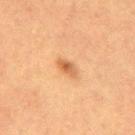Notes:
– imaging modality · ~15 mm crop, total-body skin-cancer survey
– automated metrics · a footprint of about 3 mm², a shape eccentricity near 0.9, and a shape-asymmetry score of about 0.4 (0 = symmetric); an average lesion color of about L≈49 a*≈21 b*≈34 (CIELAB) and roughly 10 lightness units darker than nearby skin; a color-variation rating of about 3.5/10; a nevus-likeness score of about 85/100 and a lesion-detection confidence of about 100/100
– size · ~3 mm (longest diameter)
– anatomic site · the back
– subject · female, aged 53–57